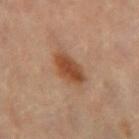Captured during whole-body skin photography for melanoma surveillance; the lesion was not biopsied.
A roughly 15 mm field-of-view crop from a total-body skin photograph.
The recorded lesion diameter is about 4.5 mm.
The subject is a female aged 48–52.
The tile uses cross-polarized illumination.
From the leg.